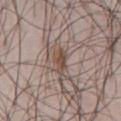Part of a total-body skin-imaging series; this lesion was reviewed on a skin check and was not flagged for biopsy. A 15 mm close-up tile from a total-body photography series done for melanoma screening. Longest diameter approximately 3.5 mm. The lesion is located on the abdomen. The subject is a male aged approximately 45. The total-body-photography lesion software estimated an area of roughly 4.5 mm², a shape eccentricity near 0.85, and a shape-asymmetry score of about 0.3 (0 = symmetric). It also reported a mean CIELAB color near L≈48 a*≈15 b*≈24, a lesion–skin lightness drop of about 10, and a lesion-to-skin contrast of about 8 (normalized; higher = more distinct). This is a white-light tile.